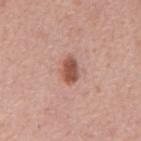body site — the mid back
patient — male, approximately 65 years of age
image — 15 mm crop, total-body photography